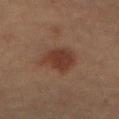The recorded lesion diameter is about 4 mm. The lesion-visualizer software estimated a lesion color around L≈32 a*≈18 b*≈24 in CIELAB, about 8 CIELAB-L* units darker than the surrounding skin, and a normalized lesion–skin contrast near 8. It also reported a border-irregularity rating of about 2.5/10 and a color-variation rating of about 2.5/10. And it measured an automated nevus-likeness rating near 100 out of 100 and lesion-presence confidence of about 100/100. The lesion is located on the abdomen. Cropped from a whole-body photographic skin survey; the tile spans about 15 mm. A female patient, aged around 60.